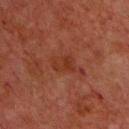Recorded during total-body skin imaging; not selected for excision or biopsy.
The tile uses cross-polarized illumination.
The lesion is located on the chest.
The recorded lesion diameter is about 3 mm.
A close-up tile cropped from a whole-body skin photograph, about 15 mm across.
A male subject in their 60s.